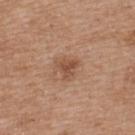{"biopsy_status": "not biopsied; imaged during a skin examination", "site": "upper back", "lesion_size": {"long_diameter_mm_approx": 2.5}, "patient": {"sex": "female", "age_approx": 40}, "image": {"source": "total-body photography crop", "field_of_view_mm": 15}, "lighting": "white-light"}